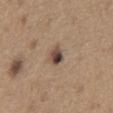Q: Was a biopsy performed?
A: total-body-photography surveillance lesion; no biopsy
Q: What kind of image is this?
A: total-body-photography crop, ~15 mm field of view
Q: Where on the body is the lesion?
A: the chest
Q: Who is the patient?
A: male, in their mid-60s